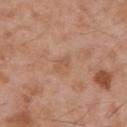Context:
A lesion tile, about 15 mm wide, cut from a 3D total-body photograph. The tile uses white-light illumination. Measured at roughly 2.5 mm in maximum diameter. Automated tile analysis of the lesion measured a border-irregularity rating of about 4/10, a color-variation rating of about 0/10, and radial color variation of about 0. And it measured a nevus-likeness score of about 0/100. The lesion is located on the upper back. A male patient aged approximately 55.